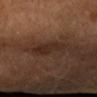{
  "lighting": "cross-polarized",
  "patient": {
    "sex": "male",
    "age_approx": 55
  },
  "automated_metrics": {
    "cielab_L": 26,
    "cielab_a": 17,
    "cielab_b": 23,
    "vs_skin_darker_L": 6.0,
    "vs_skin_contrast_norm": 7.0,
    "border_irregularity_0_10": 3.5,
    "color_variation_0_10": 2.5,
    "nevus_likeness_0_100": 0,
    "lesion_detection_confidence_0_100": 100
  },
  "lesion_size": {
    "long_diameter_mm_approx": 4.0
  },
  "site": "arm",
  "image": {
    "source": "total-body photography crop",
    "field_of_view_mm": 15
  }
}